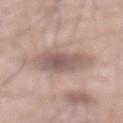Case summary:
• follow-up — catalogued during a skin exam; not biopsied
• image source — ~15 mm crop, total-body skin-cancer survey
• location — the abdomen
• patient — male, roughly 70 years of age